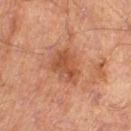The lesion was tiled from a total-body skin photograph and was not biopsied. Captured under cross-polarized illumination. The lesion's longest dimension is about 5 mm. A 15 mm close-up extracted from a 3D total-body photography capture. Automated tile analysis of the lesion measured an average lesion color of about L≈41 a*≈22 b*≈29 (CIELAB), roughly 8 lightness units darker than nearby skin, and a normalized lesion–skin contrast near 6.5. The software also gave an automated nevus-likeness rating near 35 out of 100 and a detector confidence of about 100 out of 100 that the crop contains a lesion. The lesion is located on the right thigh. A male subject roughly 70 years of age.